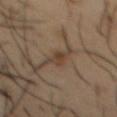Acquisition and patient details: Longest diameter approximately 2.5 mm. On the chest. The patient is a male aged approximately 55. A roughly 15 mm field-of-view crop from a total-body skin photograph.